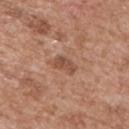Case summary:
* workup · total-body-photography surveillance lesion; no biopsy
* lighting · white-light illumination
* subject · male, aged approximately 65
* image · ~15 mm tile from a whole-body skin photo
* site · the upper back
* diameter · ~3 mm (longest diameter)